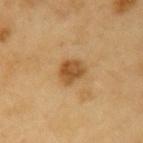<tbp_lesion>
<biopsy_status>not biopsied; imaged during a skin examination</biopsy_status>
<site>arm</site>
<patient>
  <sex>male</sex>
  <age_approx>60</age_approx>
</patient>
<image>
  <source>total-body photography crop</source>
  <field_of_view_mm>15</field_of_view_mm>
</image>
</tbp_lesion>